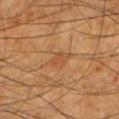No biopsy was performed on this lesion — it was imaged during a full skin examination and was not determined to be concerning. Located on the left lower leg. The recorded lesion diameter is about 2.5 mm. A 15 mm crop from a total-body photograph taken for skin-cancer surveillance. This is a cross-polarized tile. A male subject aged around 65.